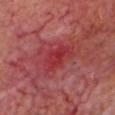| key | value |
|---|---|
| notes | no biopsy performed (imaged during a skin exam) |
| lesion size | about 4 mm |
| image-analysis metrics | an area of roughly 8.5 mm², a shape eccentricity near 0.75, and a shape-asymmetry score of about 0.3 (0 = symmetric); a border-irregularity index near 4/10, a within-lesion color-variation index near 4.5/10, and a peripheral color-asymmetry measure near 1.5; a classifier nevus-likeness of about 0/100 and a lesion-detection confidence of about 80/100 |
| subject | male, aged around 65 |
| image | 15 mm crop, total-body photography |
| anatomic site | the head or neck |